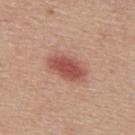biopsy status = no biopsy performed (imaged during a skin exam) | illumination = white-light illumination | subject = male, approximately 55 years of age | size = ~5 mm (longest diameter) | anatomic site = the back | image source = 15 mm crop, total-body photography.